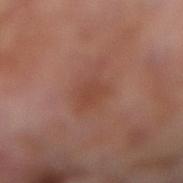Clinical impression:
Captured during whole-body skin photography for melanoma surveillance; the lesion was not biopsied.
Context:
The lesion is located on the right lower leg. Imaged with cross-polarized lighting. A 15 mm crop from a total-body photograph taken for skin-cancer surveillance. A male subject, aged approximately 70. Longest diameter approximately 6 mm.